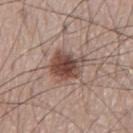This lesion was catalogued during total-body skin photography and was not selected for biopsy. The lesion-visualizer software estimated a border-irregularity index near 3/10, a color-variation rating of about 5/10, and radial color variation of about 1.5. The lesion is on the abdomen. A male subject, about 80 years old. A 15 mm crop from a total-body photograph taken for skin-cancer surveillance.